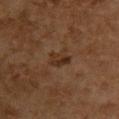<lesion>
  <biopsy_status>not biopsied; imaged during a skin examination</biopsy_status>
  <image>
    <source>total-body photography crop</source>
    <field_of_view_mm>15</field_of_view_mm>
  </image>
  <patient>
    <sex>female</sex>
    <age_approx>60</age_approx>
  </patient>
  <lesion_size>
    <long_diameter_mm_approx>2.5</long_diameter_mm_approx>
  </lesion_size>
  <site>back</site>
  <lighting>cross-polarized</lighting>
</lesion>